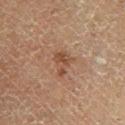| key | value |
|---|---|
| biopsy status | total-body-photography surveillance lesion; no biopsy |
| subject | female, approximately 50 years of age |
| location | the right lower leg |
| diameter | ~3 mm (longest diameter) |
| image | total-body-photography crop, ~15 mm field of view |
| lighting | cross-polarized illumination |